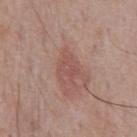This lesion was catalogued during total-body skin photography and was not selected for biopsy. The lesion is on the chest. A male patient aged approximately 70. Captured under white-light illumination. The recorded lesion diameter is about 2.5 mm. A 15 mm close-up extracted from a 3D total-body photography capture.